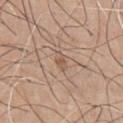| feature | finding |
|---|---|
| workup | catalogued during a skin exam; not biopsied |
| image | ~15 mm tile from a whole-body skin photo |
| subject | male, aged around 65 |
| body site | the chest |
| diameter | ≈2.5 mm |